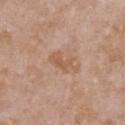Notes:
* notes · total-body-photography surveillance lesion; no biopsy
* body site · the chest
* illumination · white-light illumination
* size · about 4 mm
* patient · female, roughly 30 years of age
* acquisition · total-body-photography crop, ~15 mm field of view
* automated metrics · an area of roughly 5 mm², an outline eccentricity of about 0.9 (0 = round, 1 = elongated), and a symmetry-axis asymmetry near 0.45; about 7 CIELAB-L* units darker than the surrounding skin